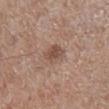This lesion was catalogued during total-body skin photography and was not selected for biopsy. This image is a 15 mm lesion crop taken from a total-body photograph. From the right lower leg. Measured at roughly 3 mm in maximum diameter. Imaged with white-light lighting. A female subject aged 73–77.